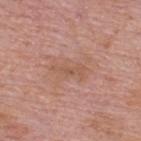notes — total-body-photography surveillance lesion; no biopsy
patient — male, aged around 75
body site — the back
imaging modality — total-body-photography crop, ~15 mm field of view
illumination — white-light illumination
lesion size — ~4 mm (longest diameter)
automated lesion analysis — a mean CIELAB color near L≈55 a*≈21 b*≈29 and a lesion-to-skin contrast of about 5 (normalized; higher = more distinct); a border-irregularity rating of about 4.5/10, a color-variation rating of about 1.5/10, and peripheral color asymmetry of about 0.5; a nevus-likeness score of about 0/100 and a detector confidence of about 100 out of 100 that the crop contains a lesion